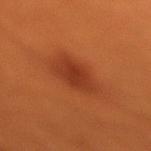Impression: The lesion was photographed on a routine skin check and not biopsied; there is no pathology result. Clinical summary: This is a cross-polarized tile. From the right lower leg. A female subject, aged 28 to 32. This image is a 15 mm lesion crop taken from a total-body photograph.